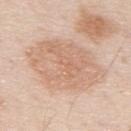Clinical impression:
This lesion was catalogued during total-body skin photography and was not selected for biopsy.
Background:
From the mid back. A male subject, in their 80s. A close-up tile cropped from a whole-body skin photograph, about 15 mm across.